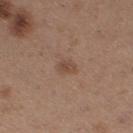Recorded during total-body skin imaging; not selected for excision or biopsy. A 15 mm close-up tile from a total-body photography series done for melanoma screening. This is a white-light tile. A female patient, about 30 years old. On the right thigh.